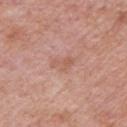This lesion was catalogued during total-body skin photography and was not selected for biopsy.
A male subject, aged 53–57.
A 15 mm close-up tile from a total-body photography series done for melanoma screening.
Longest diameter approximately 3 mm.
This is a white-light tile.
On the left upper arm.